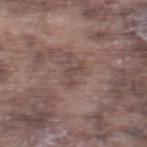size: ~3.5 mm (longest diameter) | tile lighting: white-light illumination | subject: male, about 75 years old | image source: 15 mm crop, total-body photography | TBP lesion metrics: a border-irregularity index near 8.5/10; a lesion-detection confidence of about 95/100 | anatomic site: the right thigh.